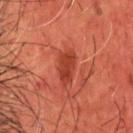No biopsy was performed on this lesion — it was imaged during a full skin examination and was not determined to be concerning.
An algorithmic analysis of the crop reported a lesion area of about 6 mm², an outline eccentricity of about 0.75 (0 = round, 1 = elongated), and two-axis asymmetry of about 0.2. The analysis additionally found roughly 8 lightness units darker than nearby skin and a lesion-to-skin contrast of about 7.5 (normalized; higher = more distinct). The analysis additionally found border irregularity of about 2 on a 0–10 scale, internal color variation of about 2 on a 0–10 scale, and radial color variation of about 0.5.
Imaged with cross-polarized lighting.
A lesion tile, about 15 mm wide, cut from a 3D total-body photograph.
The lesion's longest dimension is about 3.5 mm.
The subject is a male roughly 60 years of age.
On the head or neck.